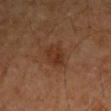  biopsy_status: not biopsied; imaged during a skin examination
  site: right upper arm
  patient:
    sex: male
    age_approx: 60
  lighting: cross-polarized
  image:
    source: total-body photography crop
    field_of_view_mm: 15
  automated_metrics:
    cielab_L: 30
    cielab_a: 20
    cielab_b: 28
    vs_skin_darker_L: 7.0
    vs_skin_contrast_norm: 7.0